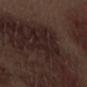follow-up: catalogued during a skin exam; not biopsied
location: the left forearm
imaging modality: ~15 mm crop, total-body skin-cancer survey
patient: male, aged around 70
lesion size: ≈6 mm
lighting: white-light illumination